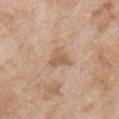This lesion was catalogued during total-body skin photography and was not selected for biopsy. Imaged with white-light lighting. A female patient aged 73 to 77. A 15 mm crop from a total-body photograph taken for skin-cancer surveillance. Located on the right upper arm.